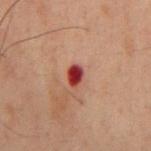Measured at roughly 2.5 mm in maximum diameter. A male patient, aged approximately 60. The total-body-photography lesion software estimated an area of roughly 3.5 mm², an eccentricity of roughly 0.8, and two-axis asymmetry of about 0.2. The software also gave a mean CIELAB color near L≈30 a*≈31 b*≈23, a lesion–skin lightness drop of about 15, and a normalized lesion–skin contrast near 14. The software also gave a within-lesion color-variation index near 3.5/10. It also reported a nevus-likeness score of about 0/100. Located on the mid back. A roughly 15 mm field-of-view crop from a total-body skin photograph.